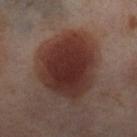• workup — catalogued during a skin exam; not biopsied
• location — the right thigh
• tile lighting — cross-polarized
• lesion size — about 8 mm
• image — ~15 mm crop, total-body skin-cancer survey
• subject — female, approximately 55 years of age